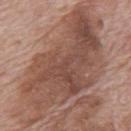Assessment:
This lesion was catalogued during total-body skin photography and was not selected for biopsy.
Clinical summary:
A male subject aged 68–72. A lesion tile, about 15 mm wide, cut from a 3D total-body photograph. This is a white-light tile. Located on the back. The recorded lesion diameter is about 16 mm.